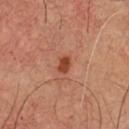This lesion was catalogued during total-body skin photography and was not selected for biopsy.
Located on the front of the torso.
Cropped from a total-body skin-imaging series; the visible field is about 15 mm.
A male subject, roughly 65 years of age.
The tile uses cross-polarized illumination.